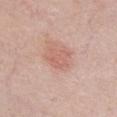Q: Was this lesion biopsied?
A: imaged on a skin check; not biopsied
Q: Where on the body is the lesion?
A: the chest
Q: Illumination type?
A: white-light illumination
Q: Automated lesion metrics?
A: a mean CIELAB color near L≈61 a*≈24 b*≈27 and a normalized lesion–skin contrast near 4.5
Q: Patient demographics?
A: female, aged 38–42
Q: How was this image acquired?
A: ~15 mm crop, total-body skin-cancer survey